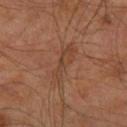Image and clinical context:
A lesion tile, about 15 mm wide, cut from a 3D total-body photograph. From the right leg. A male subject about 60 years old.